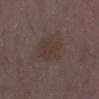<case>
  <biopsy_status>not biopsied; imaged during a skin examination</biopsy_status>
  <automated_metrics>
    <border_irregularity_0_10>2.0</border_irregularity_0_10>
    <color_variation_0_10>1.5</color_variation_0_10>
    <peripheral_color_asymmetry>0.5</peripheral_color_asymmetry>
  </automated_metrics>
  <lesion_size>
    <long_diameter_mm_approx>3.5</long_diameter_mm_approx>
  </lesion_size>
  <image>
    <source>total-body photography crop</source>
    <field_of_view_mm>15</field_of_view_mm>
  </image>
  <patient>
    <sex>female</sex>
    <age_approx>30</age_approx>
  </patient>
  <site>leg</site>
  <lighting>white-light</lighting>
</case>